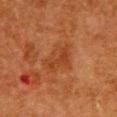Part of a total-body skin-imaging series; this lesion was reviewed on a skin check and was not flagged for biopsy. Located on the chest. Captured under cross-polarized illumination. A 15 mm crop from a total-body photograph taken for skin-cancer surveillance. Longest diameter approximately 4.5 mm. A female subject, aged approximately 50.